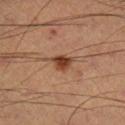Recorded during total-body skin imaging; not selected for excision or biopsy. A close-up tile cropped from a whole-body skin photograph, about 15 mm across. Imaged with cross-polarized lighting. A male subject aged around 55. Automated image analysis of the tile measured lesion-presence confidence of about 100/100. Longest diameter approximately 2.5 mm. The lesion is on the leg.